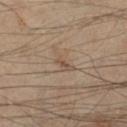- biopsy status — catalogued during a skin exam; not biopsied
- illumination — cross-polarized illumination
- image-analysis metrics — an area of roughly 3 mm², an outline eccentricity of about 0.85 (0 = round, 1 = elongated), and a symmetry-axis asymmetry near 0.35; a nevus-likeness score of about 0/100 and a detector confidence of about 95 out of 100 that the crop contains a lesion
- patient — male, aged around 55
- body site — the left lower leg
- image — total-body-photography crop, ~15 mm field of view